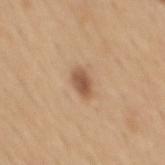Captured during whole-body skin photography for melanoma surveillance; the lesion was not biopsied. From the mid back. Measured at roughly 3 mm in maximum diameter. A lesion tile, about 15 mm wide, cut from a 3D total-body photograph. A male subject approximately 50 years of age.